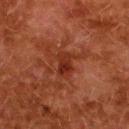Captured during whole-body skin photography for melanoma surveillance; the lesion was not biopsied. A female subject about 50 years old. A roughly 15 mm field-of-view crop from a total-body skin photograph. The tile uses cross-polarized illumination. The lesion is on the upper back. The recorded lesion diameter is about 3 mm.